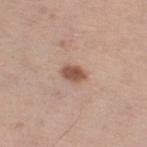Image and clinical context:
The recorded lesion diameter is about 3 mm. The lesion-visualizer software estimated a lesion area of about 4 mm² and two-axis asymmetry of about 0.15. The analysis additionally found border irregularity of about 1.5 on a 0–10 scale, a color-variation rating of about 2.5/10, and peripheral color asymmetry of about 0.5. The analysis additionally found an automated nevus-likeness rating near 90 out of 100 and a detector confidence of about 100 out of 100 that the crop contains a lesion. A female patient, in their mid- to late 60s. From the right thigh. A 15 mm close-up extracted from a 3D total-body photography capture.